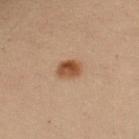notes: imaged on a skin check; not biopsied
tile lighting: cross-polarized illumination
lesion size: ~2.5 mm (longest diameter)
body site: the left forearm
image-analysis metrics: an area of roughly 5 mm², a shape eccentricity near 0.55, and a symmetry-axis asymmetry near 0.15; an automated nevus-likeness rating near 100 out of 100 and a lesion-detection confidence of about 100/100
subject: female, aged 28 to 32
image: ~15 mm crop, total-body skin-cancer survey